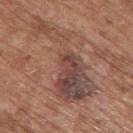Clinical summary:
About 11.5 mm across. This is a white-light tile. A male patient, about 75 years old. A lesion tile, about 15 mm wide, cut from a 3D total-body photograph. Located on the upper back.